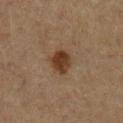Imaged during a routine full-body skin examination; the lesion was not biopsied and no histopathology is available.
The lesion is located on the left lower leg.
Cropped from a total-body skin-imaging series; the visible field is about 15 mm.
The patient is a male about 85 years old or older.
This is a cross-polarized tile.
The total-body-photography lesion software estimated a footprint of about 6 mm², an outline eccentricity of about 0.3 (0 = round, 1 = elongated), and a shape-asymmetry score of about 0.2 (0 = symmetric). It also reported an average lesion color of about L≈31 a*≈17 b*≈26 (CIELAB), about 11 CIELAB-L* units darker than the surrounding skin, and a normalized border contrast of about 10.5. The software also gave a classifier nevus-likeness of about 100/100.
The recorded lesion diameter is about 2.5 mm.